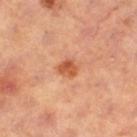<tbp_lesion>
<biopsy_status>not biopsied; imaged during a skin examination</biopsy_status>
<site>right thigh</site>
<automated_metrics>
  <cielab_L>56</cielab_L>
  <cielab_a>29</cielab_a>
  <cielab_b>39</cielab_b>
  <vs_skin_darker_L>11.0</vs_skin_darker_L>
  <vs_skin_contrast_norm>8.0</vs_skin_contrast_norm>
  <lesion_detection_confidence_0_100>100</lesion_detection_confidence_0_100>
</automated_metrics>
<patient>
  <sex>female</sex>
  <age_approx>40</age_approx>
</patient>
<image>
  <source>total-body photography crop</source>
  <field_of_view_mm>15</field_of_view_mm>
</image>
<lesion_size>
  <long_diameter_mm_approx>2.0</long_diameter_mm_approx>
</lesion_size>
</tbp_lesion>